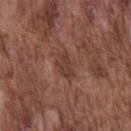Imaged during a routine full-body skin examination; the lesion was not biopsied and no histopathology is available. Located on the chest. Approximately 3.5 mm at its widest. The patient is a male aged around 75. Captured under white-light illumination. A 15 mm close-up tile from a total-body photography series done for melanoma screening.